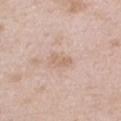<tbp_lesion>
<biopsy_status>not biopsied; imaged during a skin examination</biopsy_status>
<patient>
  <sex>male</sex>
  <age_approx>50</age_approx>
</patient>
<site>chest</site>
<image>
  <source>total-body photography crop</source>
  <field_of_view_mm>15</field_of_view_mm>
</image>
<lesion_size>
  <long_diameter_mm_approx>2.5</long_diameter_mm_approx>
</lesion_size>
<automated_metrics>
  <area_mm2_approx>3.5</area_mm2_approx>
  <eccentricity>0.85</eccentricity>
  <shape_asymmetry>0.2</shape_asymmetry>
</automated_metrics>
</tbp_lesion>